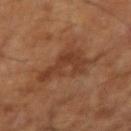Clinical summary:
Cropped from a whole-body photographic skin survey; the tile spans about 15 mm. The subject is a male approximately 65 years of age. The recorded lesion diameter is about 5 mm. The lesion is on the left arm. An algorithmic analysis of the crop reported a lesion color around L≈37 a*≈22 b*≈30 in CIELAB and roughly 8 lightness units darker than nearby skin. And it measured border irregularity of about 8.5 on a 0–10 scale, internal color variation of about 2 on a 0–10 scale, and radial color variation of about 0.5. The analysis additionally found a nevus-likeness score of about 0/100 and a lesion-detection confidence of about 100/100. The tile uses cross-polarized illumination.